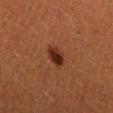Notes:
– notes — total-body-photography surveillance lesion; no biopsy
– diameter — about 2.5 mm
– lighting — cross-polarized
– image — ~15 mm crop, total-body skin-cancer survey
– automated lesion analysis — an area of roughly 4.5 mm², a shape eccentricity near 0.7, and a symmetry-axis asymmetry near 0.25; a lesion color around L≈24 a*≈21 b*≈25 in CIELAB, about 10 CIELAB-L* units darker than the surrounding skin, and a normalized border contrast of about 10.5; a border-irregularity index near 2/10 and a within-lesion color-variation index near 3.5/10; an automated nevus-likeness rating near 95 out of 100 and a detector confidence of about 100 out of 100 that the crop contains a lesion
– subject — male, aged 38–42
– location — the arm